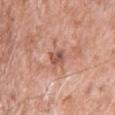{"biopsy_status": "not biopsied; imaged during a skin examination", "site": "chest", "lighting": "white-light", "patient": {"sex": "male", "age_approx": 50}, "image": {"source": "total-body photography crop", "field_of_view_mm": 15}}